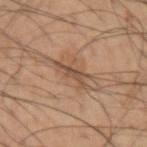{
  "biopsy_status": "not biopsied; imaged during a skin examination",
  "patient": {
    "sex": "male",
    "age_approx": 55
  },
  "site": "left upper arm",
  "image": {
    "source": "total-body photography crop",
    "field_of_view_mm": 15
  }
}